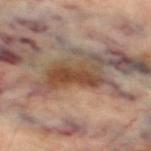A male patient aged approximately 70. Cropped from a total-body skin-imaging series; the visible field is about 15 mm. The lesion's longest dimension is about 6 mm. Automated tile analysis of the lesion measured an area of roughly 13 mm², an outline eccentricity of about 0.85 (0 = round, 1 = elongated), and a shape-asymmetry score of about 0.4 (0 = symmetric). The software also gave an average lesion color of about L≈45 a*≈18 b*≈29 (CIELAB), roughly 11 lightness units darker than nearby skin, and a lesion-to-skin contrast of about 9 (normalized; higher = more distinct). The analysis additionally found internal color variation of about 4 on a 0–10 scale. Captured under cross-polarized illumination. Located on the leg.